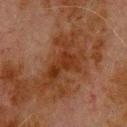biopsy status — total-body-photography surveillance lesion; no biopsy | image — ~15 mm crop, total-body skin-cancer survey | patient — male, aged around 80 | lesion size — ≈5.5 mm | location — the back | lighting — cross-polarized illumination | automated lesion analysis — a lesion area of about 9.5 mm².